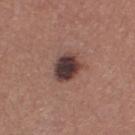Recorded during total-body skin imaging; not selected for excision or biopsy. A female subject, in their mid- to late 50s. Cropped from a whole-body photographic skin survey; the tile spans about 15 mm. This is a white-light tile. Measured at roughly 3.5 mm in maximum diameter. From the right lower leg.